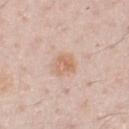Q: Is there a histopathology result?
A: total-body-photography surveillance lesion; no biopsy